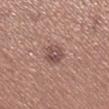Q: Was this lesion biopsied?
A: no biopsy performed (imaged during a skin exam)
Q: Lesion location?
A: the left lower leg
Q: How was the tile lit?
A: white-light illumination
Q: What is the lesion's diameter?
A: about 3 mm
Q: How was this image acquired?
A: total-body-photography crop, ~15 mm field of view
Q: What are the patient's age and sex?
A: female, in their mid-30s